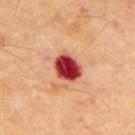Clinical impression: Imaged during a routine full-body skin examination; the lesion was not biopsied and no histopathology is available. Background: Imaged with cross-polarized lighting. Located on the upper back. Longest diameter approximately 3.5 mm. This image is a 15 mm lesion crop taken from a total-body photograph. The patient is a male about 70 years old.